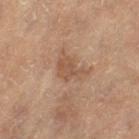Context:
The patient is a female aged 63–67. The lesion-visualizer software estimated an area of roughly 6 mm² and two-axis asymmetry of about 0.45. The software also gave border irregularity of about 5 on a 0–10 scale, internal color variation of about 2 on a 0–10 scale, and peripheral color asymmetry of about 0.5. The analysis additionally found a classifier nevus-likeness of about 0/100. A 15 mm close-up extracted from a 3D total-body photography capture. The lesion is on the left thigh.